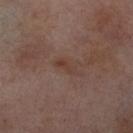follow-up = catalogued during a skin exam; not biopsied | anatomic site = the right thigh | subject = female, in their mid-50s | illumination = cross-polarized illumination | image = ~15 mm tile from a whole-body skin photo.